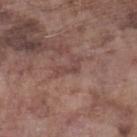Recorded during total-body skin imaging; not selected for excision or biopsy. Measured at roughly 3 mm in maximum diameter. The subject is a male aged around 75. The tile uses white-light illumination. A roughly 15 mm field-of-view crop from a total-body skin photograph. The lesion is located on the left lower leg.